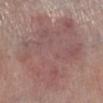Impression: No biopsy was performed on this lesion — it was imaged during a full skin examination and was not determined to be concerning. Image and clinical context: From the left lower leg. An algorithmic analysis of the crop reported an eccentricity of roughly 0.75 and a symmetry-axis asymmetry near 0.2. And it measured border irregularity of about 3.5 on a 0–10 scale and peripheral color asymmetry of about 1.5. The analysis additionally found a nevus-likeness score of about 0/100 and lesion-presence confidence of about 65/100. A male patient in their 80s. Imaged with white-light lighting. A 15 mm close-up extracted from a 3D total-body photography capture.